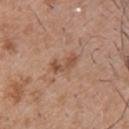lighting=white-light illumination | anatomic site=the upper back | subject=male, aged approximately 50 | image=15 mm crop, total-body photography | size=~3.5 mm (longest diameter).